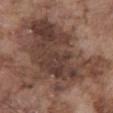workup=no biopsy performed (imaged during a skin exam)
imaging modality=total-body-photography crop, ~15 mm field of view
patient=male, aged 73–77
body site=the front of the torso
illumination=white-light
size=about 13 mm
automated metrics=a lesion area of about 65 mm² and a shape eccentricity near 0.75; an average lesion color of about L≈40 a*≈17 b*≈23 (CIELAB), a lesion–skin lightness drop of about 11, and a normalized border contrast of about 9; a detector confidence of about 55 out of 100 that the crop contains a lesion